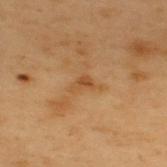Case summary:
* biopsy status — catalogued during a skin exam; not biopsied
* image — total-body-photography crop, ~15 mm field of view
* lesion diameter — about 2.5 mm
* patient — male, aged around 55
* tile lighting — cross-polarized illumination
* TBP lesion metrics — a footprint of about 3 mm² and a shape-asymmetry score of about 0.5 (0 = symmetric); peripheral color asymmetry of about 0.5; a detector confidence of about 100 out of 100 that the crop contains a lesion
* anatomic site — the upper back